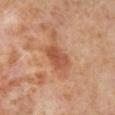The lesion was tiled from a total-body skin photograph and was not biopsied. A female subject in their mid-50s. Longest diameter approximately 3.5 mm. The lesion is located on the right lower leg. The tile uses cross-polarized illumination. Automated image analysis of the tile measured a mean CIELAB color near L≈53 a*≈27 b*≈35 and a lesion–skin lightness drop of about 10. The analysis additionally found a border-irregularity index near 2/10, a color-variation rating of about 3/10, and radial color variation of about 1. The analysis additionally found a classifier nevus-likeness of about 15/100. This image is a 15 mm lesion crop taken from a total-body photograph.